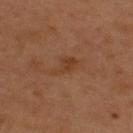No biopsy was performed on this lesion — it was imaged during a full skin examination and was not determined to be concerning.
A male patient, in their 50s.
The lesion is located on the upper back.
Imaged with cross-polarized lighting.
A 15 mm crop from a total-body photograph taken for skin-cancer surveillance.